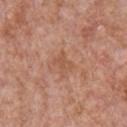The lesion was photographed on a routine skin check and not biopsied; there is no pathology result.
Longest diameter approximately 3 mm.
The total-body-photography lesion software estimated a border-irregularity index near 4.5/10, a within-lesion color-variation index near 1/10, and peripheral color asymmetry of about 0.5.
The lesion is located on the chest.
A 15 mm crop from a total-body photograph taken for skin-cancer surveillance.
A male patient, aged 63 to 67.